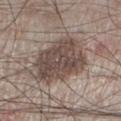Measured at roughly 6.5 mm in maximum diameter.
An algorithmic analysis of the crop reported an average lesion color of about L≈47 a*≈13 b*≈20 (CIELAB) and a normalized border contrast of about 9.5.
The lesion is located on the left lower leg.
A male subject, roughly 60 years of age.
The tile uses white-light illumination.
A 15 mm crop from a total-body photograph taken for skin-cancer surveillance.